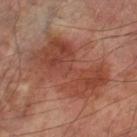Clinical impression: The lesion was tiled from a total-body skin photograph and was not biopsied. Context: From the left lower leg. This image is a 15 mm lesion crop taken from a total-body photograph. Longest diameter approximately 9 mm. An algorithmic analysis of the crop reported an area of roughly 34 mm², an eccentricity of roughly 0.85, and two-axis asymmetry of about 0.4. It also reported an average lesion color of about L≈43 a*≈25 b*≈29 (CIELAB) and a lesion-to-skin contrast of about 7.5 (normalized; higher = more distinct). The analysis additionally found a border-irregularity index near 7/10, internal color variation of about 5 on a 0–10 scale, and radial color variation of about 1.5. The analysis additionally found a lesion-detection confidence of about 100/100. The patient is a male aged 68 to 72. Imaged with cross-polarized lighting.